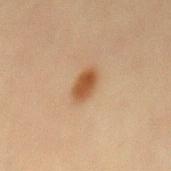Q: What are the patient's age and sex?
A: male, in their 60s
Q: Lesion location?
A: the mid back
Q: What kind of image is this?
A: ~15 mm tile from a whole-body skin photo